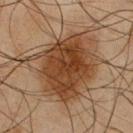Image and clinical context:
Cropped from a total-body skin-imaging series; the visible field is about 15 mm. Automated image analysis of the tile measured an area of roughly 44 mm², a shape eccentricity near 0.5, and a symmetry-axis asymmetry near 0.3. A male patient, approximately 45 years of age. Approximately 9.5 mm at its widest. From the chest.